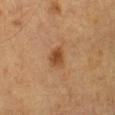{"image": {"source": "total-body photography crop", "field_of_view_mm": 15}, "patient": {"sex": "male", "age_approx": "85+"}, "site": "leg"}